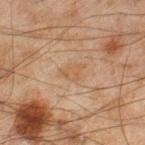No biopsy was performed on this lesion — it was imaged during a full skin examination and was not determined to be concerning.
A male patient, in their mid- to late 40s.
The recorded lesion diameter is about 2.5 mm.
From the right lower leg.
Cropped from a total-body skin-imaging series; the visible field is about 15 mm.
An algorithmic analysis of the crop reported an area of roughly 3.5 mm² and an eccentricity of roughly 0.65. The software also gave about 5 CIELAB-L* units darker than the surrounding skin. It also reported a border-irregularity rating of about 4/10, internal color variation of about 1.5 on a 0–10 scale, and radial color variation of about 0.5. It also reported a nevus-likeness score of about 0/100.
The tile uses cross-polarized illumination.